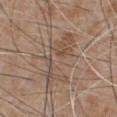Imaged during a routine full-body skin examination; the lesion was not biopsied and no histopathology is available.
A male patient approximately 65 years of age.
An algorithmic analysis of the crop reported a lesion area of about 19 mm², an outline eccentricity of about 0.85 (0 = round, 1 = elongated), and a shape-asymmetry score of about 0.45 (0 = symmetric). It also reported a border-irregularity rating of about 8/10, a within-lesion color-variation index near 5.5/10, and radial color variation of about 2. And it measured an automated nevus-likeness rating near 0 out of 100 and lesion-presence confidence of about 90/100.
Measured at roughly 7 mm in maximum diameter.
Cropped from a total-body skin-imaging series; the visible field is about 15 mm.
The lesion is on the chest.